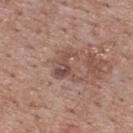notes: catalogued during a skin exam; not biopsied | location: the upper back | lighting: white-light illumination | automated metrics: a lesion color around L≈48 a*≈20 b*≈24 in CIELAB, about 9 CIELAB-L* units darker than the surrounding skin, and a normalized lesion–skin contrast near 7 | subject: male, in their 70s | imaging modality: 15 mm crop, total-body photography.